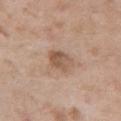  biopsy_status: not biopsied; imaged during a skin examination
  site: arm
  patient:
    sex: male
    age_approx: 50
  image:
    source: total-body photography crop
    field_of_view_mm: 15
  lighting: white-light